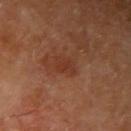Q: Was this lesion biopsied?
A: imaged on a skin check; not biopsied
Q: Where on the body is the lesion?
A: the right upper arm
Q: Patient demographics?
A: male, in their 70s
Q: What kind of image is this?
A: ~15 mm crop, total-body skin-cancer survey